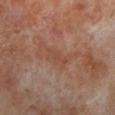This lesion was catalogued during total-body skin photography and was not selected for biopsy. Longest diameter approximately 3.5 mm. A 15 mm crop from a total-body photograph taken for skin-cancer surveillance. Imaged with cross-polarized lighting. The lesion is on the left lower leg. The lesion-visualizer software estimated a mean CIELAB color near L≈45 a*≈21 b*≈28, about 6 CIELAB-L* units darker than the surrounding skin, and a lesion-to-skin contrast of about 5 (normalized; higher = more distinct). It also reported border irregularity of about 5.5 on a 0–10 scale, a color-variation rating of about 0.5/10, and radial color variation of about 0. The patient is a male in their 70s.